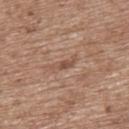biopsy status: imaged on a skin check; not biopsied.